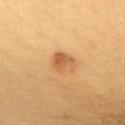Q: Was this lesion biopsied?
A: total-body-photography surveillance lesion; no biopsy
Q: How large is the lesion?
A: ~2.5 mm (longest diameter)
Q: Lesion location?
A: the upper back
Q: What is the imaging modality?
A: 15 mm crop, total-body photography
Q: What are the patient's age and sex?
A: male, aged 53 to 57